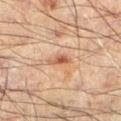Impression: Part of a total-body skin-imaging series; this lesion was reviewed on a skin check and was not flagged for biopsy. Context: A male patient, approximately 60 years of age. A roughly 15 mm field-of-view crop from a total-body skin photograph. The total-body-photography lesion software estimated a footprint of about 3 mm², an outline eccentricity of about 0.8 (0 = round, 1 = elongated), and a shape-asymmetry score of about 0.4 (0 = symmetric). The software also gave a mean CIELAB color near L≈43 a*≈19 b*≈27 and a lesion-to-skin contrast of about 7.5 (normalized; higher = more distinct). And it measured a border-irregularity index near 4/10, a within-lesion color-variation index near 3/10, and a peripheral color-asymmetry measure near 1. The lesion is on the left lower leg. The tile uses cross-polarized illumination. About 2.5 mm across.